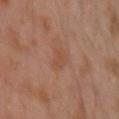Impression:
This lesion was catalogued during total-body skin photography and was not selected for biopsy.
Image and clinical context:
Longest diameter approximately 3.5 mm. An algorithmic analysis of the crop reported an outline eccentricity of about 0.85 (0 = round, 1 = elongated) and a shape-asymmetry score of about 0.3 (0 = symmetric). And it measured a border-irregularity index near 3/10, internal color variation of about 1.5 on a 0–10 scale, and radial color variation of about 0.5. The patient is a male in their 30s. Located on the left upper arm. A lesion tile, about 15 mm wide, cut from a 3D total-body photograph. The tile uses cross-polarized illumination.